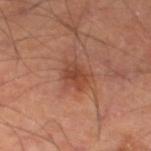| feature | finding |
|---|---|
| notes | catalogued during a skin exam; not biopsied |
| body site | the left thigh |
| diameter | about 3 mm |
| image-analysis metrics | an automated nevus-likeness rating near 80 out of 100 and a lesion-detection confidence of about 100/100 |
| image source | ~15 mm tile from a whole-body skin photo |